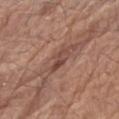{
  "biopsy_status": "not biopsied; imaged during a skin examination",
  "automated_metrics": {
    "area_mm2_approx": 4.0,
    "eccentricity": 0.95,
    "shape_asymmetry": 0.4,
    "border_irregularity_0_10": 5.0,
    "color_variation_0_10": 0.0,
    "nevus_likeness_0_100": 0
  },
  "site": "arm",
  "lighting": "white-light",
  "lesion_size": {
    "long_diameter_mm_approx": 3.5
  },
  "image": {
    "source": "total-body photography crop",
    "field_of_view_mm": 15
  },
  "patient": {
    "sex": "male",
    "age_approx": 70
  }
}